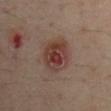| field | value |
|---|---|
| subject | male, in their mid- to late 40s |
| size | about 5 mm |
| body site | the arm |
| image source | total-body-photography crop, ~15 mm field of view |